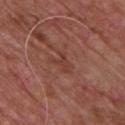Notes:
* biopsy status · no biopsy performed (imaged during a skin exam)
* imaging modality · ~15 mm tile from a whole-body skin photo
* body site · the front of the torso
* patient · male, in their mid- to late 70s
* lighting · white-light illumination